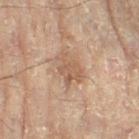Case summary:
- notes: catalogued during a skin exam; not biopsied
- anatomic site: the right leg
- patient: female, roughly 80 years of age
- lesion size: ~4 mm (longest diameter)
- acquisition: ~15 mm crop, total-body skin-cancer survey